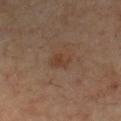Impression:
Part of a total-body skin-imaging series; this lesion was reviewed on a skin check and was not flagged for biopsy.
Background:
A male subject aged 68 to 72. A 15 mm close-up tile from a total-body photography series done for melanoma screening. This is a cross-polarized tile. On the chest. The lesion's longest dimension is about 2.5 mm.